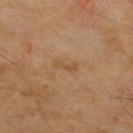Assessment:
Imaged during a routine full-body skin examination; the lesion was not biopsied and no histopathology is available.
Image and clinical context:
A male patient aged around 55. Captured under cross-polarized illumination. An algorithmic analysis of the crop reported a mean CIELAB color near L≈46 a*≈17 b*≈34, about 6 CIELAB-L* units darker than the surrounding skin, and a normalized border contrast of about 5.5. The software also gave border irregularity of about 4.5 on a 0–10 scale, internal color variation of about 0 on a 0–10 scale, and radial color variation of about 0. On the upper back. About 3 mm across. A 15 mm close-up tile from a total-body photography series done for melanoma screening.